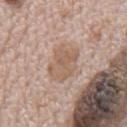Part of a total-body skin-imaging series; this lesion was reviewed on a skin check and was not flagged for biopsy. The tile uses white-light illumination. Automated tile analysis of the lesion measured a footprint of about 12 mm² and an eccentricity of roughly 0.75. And it measured a lesion color around L≈59 a*≈17 b*≈28 in CIELAB and about 8 CIELAB-L* units darker than the surrounding skin. The lesion is on the mid back. The subject is a male aged approximately 70. Cropped from a total-body skin-imaging series; the visible field is about 15 mm.